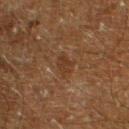notes: catalogued during a skin exam; not biopsied | patient: male, approximately 60 years of age | anatomic site: the left lower leg | acquisition: ~15 mm crop, total-body skin-cancer survey | image-analysis metrics: an area of roughly 3.5 mm² and a symmetry-axis asymmetry near 0.3; a border-irregularity rating of about 3/10, a within-lesion color-variation index near 1/10, and radial color variation of about 0.5.